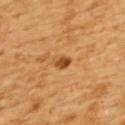Captured during whole-body skin photography for melanoma surveillance; the lesion was not biopsied. A female patient aged approximately 55. Captured under cross-polarized illumination. Cropped from a whole-body photographic skin survey; the tile spans about 15 mm. From the upper back.